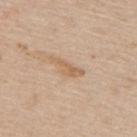Recorded during total-body skin imaging; not selected for excision or biopsy.
About 4 mm across.
A close-up tile cropped from a whole-body skin photograph, about 15 mm across.
Located on the upper back.
The lesion-visualizer software estimated border irregularity of about 5.5 on a 0–10 scale, a color-variation rating of about 1/10, and a peripheral color-asymmetry measure near 0.5. The analysis additionally found a nevus-likeness score of about 0/100 and a detector confidence of about 100 out of 100 that the crop contains a lesion.
This is a white-light tile.
A male patient, in their mid-60s.